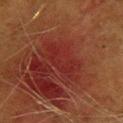The lesion was photographed on a routine skin check and not biopsied; there is no pathology result. Cropped from a whole-body photographic skin survey; the tile spans about 15 mm. Located on the upper back. The subject is a female aged 38 to 42.